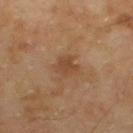The lesion was photographed on a routine skin check and not biopsied; there is no pathology result.
Captured under cross-polarized illumination.
The lesion is located on the upper back.
A 15 mm close-up tile from a total-body photography series done for melanoma screening.
A male subject, aged approximately 55.
Approximately 3 mm at its widest.